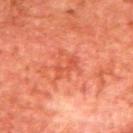size: about 3 mm | image source: total-body-photography crop, ~15 mm field of view | patient: male, about 65 years old | anatomic site: the back.